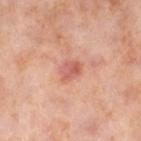Captured during whole-body skin photography for melanoma surveillance; the lesion was not biopsied.
About 3 mm across.
Cropped from a total-body skin-imaging series; the visible field is about 15 mm.
A female patient aged around 55.
On the right lower leg.